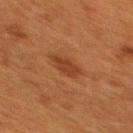Imaged during a routine full-body skin examination; the lesion was not biopsied and no histopathology is available.
The lesion is on the back.
The lesion-visualizer software estimated a border-irregularity rating of about 2.5/10.
This image is a 15 mm lesion crop taken from a total-body photograph.
About 3 mm across.
Captured under cross-polarized illumination.
The subject is a female aged 38–42.